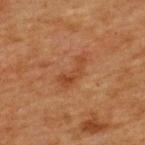The lesion was tiled from a total-body skin photograph and was not biopsied. The recorded lesion diameter is about 4 mm. This is a cross-polarized tile. A region of skin cropped from a whole-body photographic capture, roughly 15 mm wide. The patient is a female roughly 40 years of age. The lesion is located on the upper back.